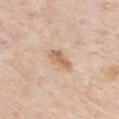This lesion was catalogued during total-body skin photography and was not selected for biopsy. An algorithmic analysis of the crop reported a lesion area of about 4.5 mm², a shape eccentricity near 0.9, and a symmetry-axis asymmetry near 0.35. And it measured a lesion color around L≈64 a*≈18 b*≈34 in CIELAB, about 10 CIELAB-L* units darker than the surrounding skin, and a normalized lesion–skin contrast near 7.5. It also reported a nevus-likeness score of about 15/100. The lesion is located on the chest. Imaged with white-light lighting. The subject is a male about 70 years old. A region of skin cropped from a whole-body photographic capture, roughly 15 mm wide. Longest diameter approximately 3.5 mm.